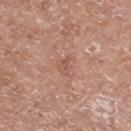Clinical impression: Imaged during a routine full-body skin examination; the lesion was not biopsied and no histopathology is available. Clinical summary: Approximately 2.5 mm at its widest. Located on the right lower leg. Imaged with white-light lighting. Cropped from a whole-body photographic skin survey; the tile spans about 15 mm. A male patient, aged 58–62.